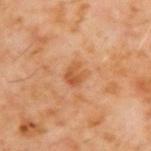imaging modality = total-body-photography crop, ~15 mm field of view
location = the upper back
patient = male, aged approximately 60